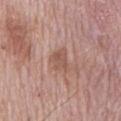Clinical impression:
Recorded during total-body skin imaging; not selected for excision or biopsy.
Context:
A lesion tile, about 15 mm wide, cut from a 3D total-body photograph. Longest diameter approximately 3 mm. On the mid back. The subject is a male aged 73–77.